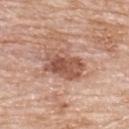This lesion was catalogued during total-body skin photography and was not selected for biopsy.
The lesion-visualizer software estimated a footprint of about 13 mm², a shape eccentricity near 0.8, and two-axis asymmetry of about 0.25. The analysis additionally found border irregularity of about 3 on a 0–10 scale, a within-lesion color-variation index near 5.5/10, and radial color variation of about 2. The analysis additionally found a classifier nevus-likeness of about 5/100.
Imaged with white-light lighting.
Measured at roughly 5 mm in maximum diameter.
From the upper back.
A roughly 15 mm field-of-view crop from a total-body skin photograph.
A male patient, aged 78 to 82.